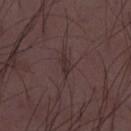Part of a total-body skin-imaging series; this lesion was reviewed on a skin check and was not flagged for biopsy.
The patient is a male aged 48–52.
An algorithmic analysis of the crop reported a lesion color around L≈29 a*≈15 b*≈15 in CIELAB and a normalized lesion–skin contrast near 6.5. And it measured a border-irregularity index near 3/10, internal color variation of about 1 on a 0–10 scale, and peripheral color asymmetry of about 0.5.
Captured under white-light illumination.
A close-up tile cropped from a whole-body skin photograph, about 15 mm across.